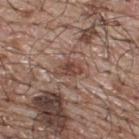size: about 3.5 mm; lighting: white-light illumination; acquisition: ~15 mm crop, total-body skin-cancer survey; subject: male, aged approximately 65; body site: the upper back.